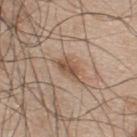body site = the upper back
imaging modality = 15 mm crop, total-body photography
patient = male, about 45 years old
lesion size = ≈3.5 mm
tile lighting = white-light